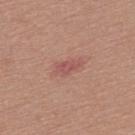Located on the upper back.
The lesion's longest dimension is about 4 mm.
Automated image analysis of the tile measured an area of roughly 5 mm², a shape eccentricity near 0.9, and two-axis asymmetry of about 0.3. And it measured border irregularity of about 3.5 on a 0–10 scale.
The tile uses white-light illumination.
A close-up tile cropped from a whole-body skin photograph, about 15 mm across.
A male subject aged around 55.